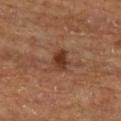workup: imaged on a skin check; not biopsied
image source: 15 mm crop, total-body photography
lesion size: about 3 mm
tile lighting: cross-polarized illumination
location: the upper back
subject: male, about 65 years old